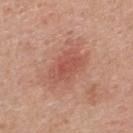Q: Was a biopsy performed?
A: total-body-photography surveillance lesion; no biopsy
Q: What is the anatomic site?
A: the upper back
Q: What is the imaging modality?
A: total-body-photography crop, ~15 mm field of view
Q: What is the lesion's diameter?
A: ≈4.5 mm
Q: What are the patient's age and sex?
A: male, aged around 55
Q: What did automated image analysis measure?
A: an area of roughly 9.5 mm² and a shape-asymmetry score of about 0.25 (0 = symmetric); a border-irregularity index near 3/10, internal color variation of about 2.5 on a 0–10 scale, and radial color variation of about 1